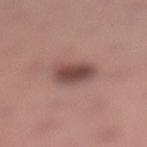illumination: white-light illumination; location: the left lower leg; image source: ~15 mm crop, total-body skin-cancer survey; diameter: ~3.5 mm (longest diameter); subject: female, aged 53 to 57.